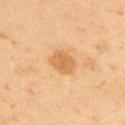A 15 mm close-up tile from a total-body photography series done for melanoma screening.
About 3 mm across.
The lesion is on the upper back.
Automated image analysis of the tile measured a footprint of about 6.5 mm², an outline eccentricity of about 0.55 (0 = round, 1 = elongated), and two-axis asymmetry of about 0.15. The analysis additionally found an average lesion color of about L≈52 a*≈18 b*≈36 (CIELAB) and a lesion–skin lightness drop of about 8. The software also gave a border-irregularity index near 1.5/10, internal color variation of about 2 on a 0–10 scale, and radial color variation of about 0.5.
A male subject, aged approximately 45.
This is a cross-polarized tile.